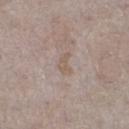• workup — imaged on a skin check; not biopsied
• patient — female, aged around 40
• image — ~15 mm tile from a whole-body skin photo
• size — about 2.5 mm
• tile lighting — white-light
• anatomic site — the left lower leg